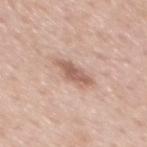Part of a total-body skin-imaging series; this lesion was reviewed on a skin check and was not flagged for biopsy. The total-body-photography lesion software estimated a lesion area of about 6.5 mm², a shape eccentricity near 0.9, and a symmetry-axis asymmetry near 0.25. And it measured a border-irregularity index near 3/10, a color-variation rating of about 2.5/10, and peripheral color asymmetry of about 1. And it measured a classifier nevus-likeness of about 20/100 and a detector confidence of about 100 out of 100 that the crop contains a lesion. Measured at roughly 4 mm in maximum diameter. A male subject, about 60 years old. This is a white-light tile. The lesion is located on the mid back. A 15 mm close-up extracted from a 3D total-body photography capture.